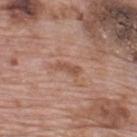Q: Is there a histopathology result?
A: catalogued during a skin exam; not biopsied
Q: Lesion size?
A: ≈3 mm
Q: What did automated image analysis measure?
A: a shape eccentricity near 0.95; a lesion color around L≈52 a*≈22 b*≈29 in CIELAB and roughly 9 lightness units darker than nearby skin; border irregularity of about 3.5 on a 0–10 scale and internal color variation of about 0 on a 0–10 scale
Q: What kind of image is this?
A: ~15 mm tile from a whole-body skin photo
Q: What lighting was used for the tile?
A: white-light illumination
Q: What is the anatomic site?
A: the upper back
Q: Who is the patient?
A: male, approximately 70 years of age